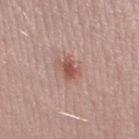This lesion was catalogued during total-body skin photography and was not selected for biopsy. A 15 mm close-up extracted from a 3D total-body photography capture. Longest diameter approximately 2.5 mm. Located on the left thigh. This is a white-light tile. A female patient aged 23 to 27.